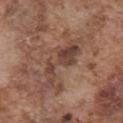Notes:
• follow-up — imaged on a skin check; not biopsied